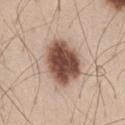Case summary:
• workup: imaged on a skin check; not biopsied
• lighting: white-light
• subject: male, aged 43–47
• image source: 15 mm crop, total-body photography
• body site: the abdomen